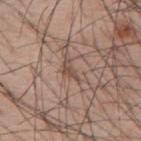Assessment:
The lesion was photographed on a routine skin check and not biopsied; there is no pathology result.